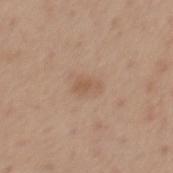notes: imaged on a skin check; not biopsied